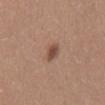Notes:
– notes · no biopsy performed (imaged during a skin exam)
– lesion diameter · about 2.5 mm
– automated metrics · a lesion color around L≈47 a*≈20 b*≈26 in CIELAB, about 11 CIELAB-L* units darker than the surrounding skin, and a normalized border contrast of about 8.5; a nevus-likeness score of about 100/100
– location · the lower back
– tile lighting · white-light illumination
– subject · female, about 35 years old
– acquisition · 15 mm crop, total-body photography